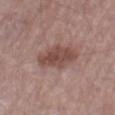Imaged during a routine full-body skin examination; the lesion was not biopsied and no histopathology is available. A 15 mm close-up extracted from a 3D total-body photography capture. The patient is a male aged around 70. Located on the right thigh.